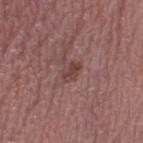Q: Is there a histopathology result?
A: total-body-photography surveillance lesion; no biopsy
Q: Where on the body is the lesion?
A: the left thigh
Q: Who is the patient?
A: female, aged 43–47
Q: Lesion size?
A: ~3 mm (longest diameter)
Q: What kind of image is this?
A: total-body-photography crop, ~15 mm field of view
Q: Automated lesion metrics?
A: a color-variation rating of about 2/10
Q: What lighting was used for the tile?
A: white-light illumination